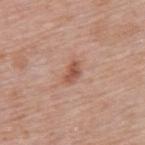{
  "biopsy_status": "not biopsied; imaged during a skin examination",
  "image": {
    "source": "total-body photography crop",
    "field_of_view_mm": 15
  },
  "automated_metrics": {
    "area_mm2_approx": 3.5,
    "eccentricity": 0.8,
    "shape_asymmetry": 0.3,
    "border_irregularity_0_10": 2.5,
    "peripheral_color_asymmetry": 0.5,
    "nevus_likeness_0_100": 60,
    "lesion_detection_confidence_0_100": 100
  },
  "patient": {
    "sex": "female",
    "age_approx": 60
  },
  "site": "upper back",
  "lesion_size": {
    "long_diameter_mm_approx": 2.5
  },
  "lighting": "white-light"
}